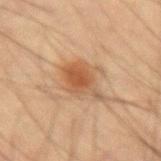Q: Is there a histopathology result?
A: no biopsy performed (imaged during a skin exam)
Q: What is the lesion's diameter?
A: ≈4.5 mm
Q: How was this image acquired?
A: 15 mm crop, total-body photography
Q: Who is the patient?
A: male, approximately 55 years of age
Q: Where on the body is the lesion?
A: the right forearm